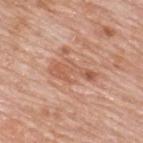| key | value |
|---|---|
| biopsy status | no biopsy performed (imaged during a skin exam) |